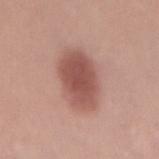biopsy status: total-body-photography surveillance lesion; no biopsy
subject: female, aged 23–27
image source: total-body-photography crop, ~15 mm field of view
lesion diameter: ≈6 mm
TBP lesion metrics: a footprint of about 19 mm², an eccentricity of roughly 0.8, and a symmetry-axis asymmetry near 0.1; a lesion color around L≈52 a*≈24 b*≈25 in CIELAB and a normalized border contrast of about 8.5; a border-irregularity rating of about 1/10, a within-lesion color-variation index near 3.5/10, and peripheral color asymmetry of about 1
illumination: white-light illumination
anatomic site: the back